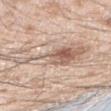Q: Was a biopsy performed?
A: catalogued during a skin exam; not biopsied
Q: What are the patient's age and sex?
A: male, roughly 25 years of age
Q: Illumination type?
A: white-light illumination
Q: Lesion location?
A: the right forearm
Q: How was this image acquired?
A: 15 mm crop, total-body photography
Q: What is the lesion's diameter?
A: ≈10 mm